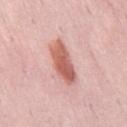<tbp_lesion>
  <biopsy_status>not biopsied; imaged during a skin examination</biopsy_status>
  <image>
    <source>total-body photography crop</source>
    <field_of_view_mm>15</field_of_view_mm>
  </image>
  <lighting>white-light</lighting>
  <site>lower back</site>
  <patient>
    <sex>male</sex>
    <age_approx>55</age_approx>
  </patient>
  <lesion_size>
    <long_diameter_mm_approx>6.0</long_diameter_mm_approx>
  </lesion_size>
</tbp_lesion>